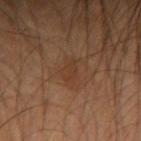<tbp_lesion>
<biopsy_status>not biopsied; imaged during a skin examination</biopsy_status>
<image>
  <source>total-body photography crop</source>
  <field_of_view_mm>15</field_of_view_mm>
</image>
<site>right forearm</site>
<automated_metrics>
  <color_variation_0_10>1.0</color_variation_0_10>
</automated_metrics>
<lesion_size>
  <long_diameter_mm_approx>2.5</long_diameter_mm_approx>
</lesion_size>
<patient>
  <sex>male</sex>
  <age_approx>55</age_approx>
</patient>
</tbp_lesion>